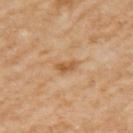No biopsy was performed on this lesion — it was imaged during a full skin examination and was not determined to be concerning.
Cropped from a whole-body photographic skin survey; the tile spans about 15 mm.
The patient is a female in their 60s.
Imaged with cross-polarized lighting.
On the arm.
The recorded lesion diameter is about 2.5 mm.